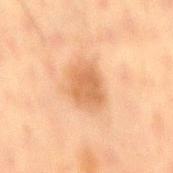  biopsy_status: not biopsied; imaged during a skin examination
  site: mid back
  patient:
    sex: male
    age_approx: 50
  image:
    source: total-body photography crop
    field_of_view_mm: 15